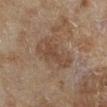The lesion was tiled from a total-body skin photograph and was not biopsied. From the right lower leg. This is a cross-polarized tile. This image is a 15 mm lesion crop taken from a total-body photograph. Longest diameter approximately 5.5 mm. A female subject, aged 58 to 62.